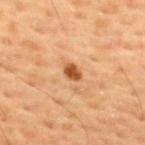Imaged during a routine full-body skin examination; the lesion was not biopsied and no histopathology is available. On the upper back. Approximately 2.5 mm at its widest. The tile uses cross-polarized illumination. A male patient in their mid-50s. This image is a 15 mm lesion crop taken from a total-body photograph.